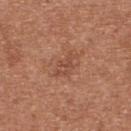<tbp_lesion>
<image>
  <source>total-body photography crop</source>
  <field_of_view_mm>15</field_of_view_mm>
</image>
<lighting>white-light</lighting>
<site>upper back</site>
<automated_metrics>
  <cielab_L>49</cielab_L>
  <cielab_a>24</cielab_a>
  <cielab_b>31</cielab_b>
  <vs_skin_darker_L>7.0</vs_skin_darker_L>
  <vs_skin_contrast_norm>5.0</vs_skin_contrast_norm>
  <border_irregularity_0_10>4.0</border_irregularity_0_10>
  <peripheral_color_asymmetry>1.0</peripheral_color_asymmetry>
  <nevus_likeness_0_100>0</nevus_likeness_0_100>
  <lesion_detection_confidence_0_100>100</lesion_detection_confidence_0_100>
</automated_metrics>
<patient>
  <sex>female</sex>
  <age_approx>40</age_approx>
</patient>
</tbp_lesion>